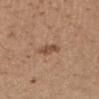follow-up = no biopsy performed (imaged during a skin exam)
image = total-body-photography crop, ~15 mm field of view
diameter = ~2.5 mm (longest diameter)
lighting = white-light illumination
site = the right upper arm
subject = female, aged around 30
image-analysis metrics = a nevus-likeness score of about 80/100 and a detector confidence of about 100 out of 100 that the crop contains a lesion